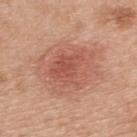Part of a total-body skin-imaging series; this lesion was reviewed on a skin check and was not flagged for biopsy.
Automated tile analysis of the lesion measured about 9 CIELAB-L* units darker than the surrounding skin.
A male subject aged around 70.
The lesion is located on the back.
A region of skin cropped from a whole-body photographic capture, roughly 15 mm wide.
The recorded lesion diameter is about 7.5 mm.
This is a white-light tile.